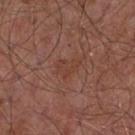Imaged during a routine full-body skin examination; the lesion was not biopsied and no histopathology is available. The lesion is on the chest. A 15 mm close-up extracted from a 3D total-body photography capture. A male patient aged approximately 55. Captured under white-light illumination. The total-body-photography lesion software estimated a lesion area of about 5 mm² and an eccentricity of roughly 0.7. And it measured a border-irregularity index near 4/10, a color-variation rating of about 2.5/10, and a peripheral color-asymmetry measure near 1. It also reported an automated nevus-likeness rating near 0 out of 100 and lesion-presence confidence of about 100/100.